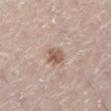Q: Is there a histopathology result?
A: catalogued during a skin exam; not biopsied
Q: What kind of image is this?
A: total-body-photography crop, ~15 mm field of view
Q: Illumination type?
A: white-light
Q: Where on the body is the lesion?
A: the right lower leg
Q: What is the lesion's diameter?
A: ≈2.5 mm
Q: Patient demographics?
A: female, aged 38–42
Q: What did automated image analysis measure?
A: a lesion color around L≈56 a*≈18 b*≈27 in CIELAB, about 11 CIELAB-L* units darker than the surrounding skin, and a normalized lesion–skin contrast near 8; an automated nevus-likeness rating near 65 out of 100 and lesion-presence confidence of about 100/100